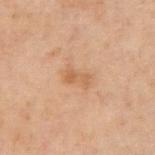Case summary:
- biopsy status: catalogued during a skin exam; not biopsied
- lighting: cross-polarized illumination
- subject: male, roughly 70 years of age
- anatomic site: the abdomen
- image: ~15 mm crop, total-body skin-cancer survey
- automated lesion analysis: a lesion color around L≈45 a*≈17 b*≈29 in CIELAB and a lesion–skin lightness drop of about 6
- size: ≈2.5 mm